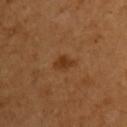Notes:
- workup — catalogued during a skin exam; not biopsied
- acquisition — ~15 mm crop, total-body skin-cancer survey
- site — the right upper arm
- illumination — cross-polarized
- subject — female, aged 53 to 57